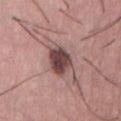Impression: No biopsy was performed on this lesion — it was imaged during a full skin examination and was not determined to be concerning. Context: A female patient, in their mid-30s. A close-up tile cropped from a whole-body skin photograph, about 15 mm across. Measured at roughly 4 mm in maximum diameter. Located on the abdomen.